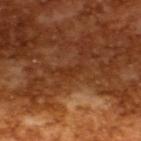Findings:
- notes · imaged on a skin check; not biopsied